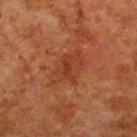biopsy status: total-body-photography surveillance lesion; no biopsy